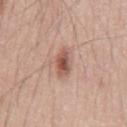Q: Is there a histopathology result?
A: total-body-photography surveillance lesion; no biopsy
Q: What are the patient's age and sex?
A: male, aged around 55
Q: What kind of image is this?
A: 15 mm crop, total-body photography
Q: Lesion size?
A: ≈3.5 mm
Q: Illumination type?
A: white-light illumination
Q: Where on the body is the lesion?
A: the chest